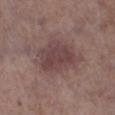Captured during whole-body skin photography for melanoma surveillance; the lesion was not biopsied.
From the left lower leg.
The tile uses white-light illumination.
A female patient, aged approximately 85 or older.
Longest diameter approximately 6 mm.
The total-body-photography lesion software estimated radial color variation of about 1.
A lesion tile, about 15 mm wide, cut from a 3D total-body photograph.